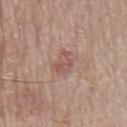Assessment:
No biopsy was performed on this lesion — it was imaged during a full skin examination and was not determined to be concerning.
Acquisition and patient details:
Located on the chest. The tile uses white-light illumination. A male patient in their 80s. A region of skin cropped from a whole-body photographic capture, roughly 15 mm wide.